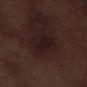workup: catalogued during a skin exam; not biopsied | image: ~15 mm crop, total-body skin-cancer survey | location: the right lower leg | patient: male, in their 70s.